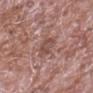Clinical impression:
Part of a total-body skin-imaging series; this lesion was reviewed on a skin check and was not flagged for biopsy.
Background:
The lesion is located on the right forearm. The tile uses white-light illumination. A male subject, about 75 years old. This image is a 15 mm lesion crop taken from a total-body photograph.